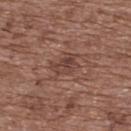biopsy_status: not biopsied; imaged during a skin examination